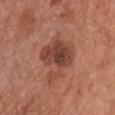Imaged during a routine full-body skin examination; the lesion was not biopsied and no histopathology is available.
The lesion's longest dimension is about 6 mm.
The lesion is on the chest.
A 15 mm crop from a total-body photograph taken for skin-cancer surveillance.
The lesion-visualizer software estimated a mean CIELAB color near L≈45 a*≈25 b*≈29, a lesion–skin lightness drop of about 10, and a lesion-to-skin contrast of about 7.5 (normalized; higher = more distinct).
A female subject, aged 63 to 67.